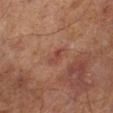Captured during whole-body skin photography for melanoma surveillance; the lesion was not biopsied. The subject is a male aged 68 to 72. Automated tile analysis of the lesion measured a footprint of about 2.5 mm². The software also gave an automated nevus-likeness rating near 0 out of 100 and a detector confidence of about 100 out of 100 that the crop contains a lesion. Located on the left lower leg. A 15 mm crop from a total-body photograph taken for skin-cancer surveillance.